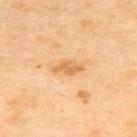Assessment:
The lesion was tiled from a total-body skin photograph and was not biopsied.
Image and clinical context:
A roughly 15 mm field-of-view crop from a total-body skin photograph. The patient is a female aged around 40. This is a cross-polarized tile. Located on the back. Measured at roughly 3.5 mm in maximum diameter.